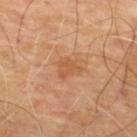Q: Was this lesion biopsied?
A: catalogued during a skin exam; not biopsied
Q: Lesion location?
A: the upper back
Q: Who is the patient?
A: male, roughly 65 years of age
Q: Illumination type?
A: cross-polarized
Q: How large is the lesion?
A: about 3 mm
Q: What is the imaging modality?
A: ~15 mm crop, total-body skin-cancer survey